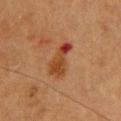No biopsy was performed on this lesion — it was imaged during a full skin examination and was not determined to be concerning.
A 15 mm crop from a total-body photograph taken for skin-cancer surveillance.
The subject is a female in their 60s.
About 4.5 mm across.
On the upper back.
Automated image analysis of the tile measured a lesion area of about 7.5 mm², a shape eccentricity near 0.9, and two-axis asymmetry of about 0.4. And it measured an average lesion color of about L≈39 a*≈24 b*≈34 (CIELAB), about 10 CIELAB-L* units darker than the surrounding skin, and a normalized lesion–skin contrast near 8.5. The software also gave a border-irregularity index near 5/10, internal color variation of about 6 on a 0–10 scale, and a peripheral color-asymmetry measure near 1.5. It also reported a nevus-likeness score of about 0/100 and a lesion-detection confidence of about 100/100.